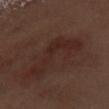No biopsy was performed on this lesion — it was imaged during a full skin examination and was not determined to be concerning. From the arm. This image is a 15 mm lesion crop taken from a total-body photograph. Captured under white-light illumination. A male subject, aged around 70.